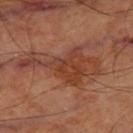The lesion was photographed on a routine skin check and not biopsied; there is no pathology result.
The tile uses cross-polarized illumination.
An algorithmic analysis of the crop reported an area of roughly 20 mm² and a shape-asymmetry score of about 0.6 (0 = symmetric). It also reported a classifier nevus-likeness of about 5/100 and a detector confidence of about 100 out of 100 that the crop contains a lesion.
Cropped from a whole-body photographic skin survey; the tile spans about 15 mm.
Longest diameter approximately 9.5 mm.
From the right thigh.
The subject is aged around 65.